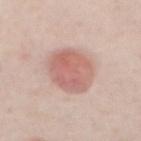The lesion was photographed on a routine skin check and not biopsied; there is no pathology result.
The subject is a male aged around 40.
A 15 mm crop from a total-body photograph taken for skin-cancer surveillance.
An algorithmic analysis of the crop reported a lesion area of about 19 mm², an eccentricity of roughly 0.65, and a shape-asymmetry score of about 0.1 (0 = symmetric). The software also gave border irregularity of about 1 on a 0–10 scale, a within-lesion color-variation index near 4.5/10, and peripheral color asymmetry of about 1.5. And it measured a classifier nevus-likeness of about 100/100 and a lesion-detection confidence of about 100/100.
On the abdomen.